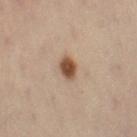Clinical impression: The lesion was tiled from a total-body skin photograph and was not biopsied. Clinical summary: The lesion's longest dimension is about 2.5 mm. The lesion is on the left lower leg. A female subject, in their 50s. A close-up tile cropped from a whole-body skin photograph, about 15 mm across.